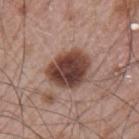This lesion was catalogued during total-body skin photography and was not selected for biopsy. This is a white-light tile. The lesion is located on the arm. The total-body-photography lesion software estimated a footprint of about 16 mm², an eccentricity of roughly 0.6, and a shape-asymmetry score of about 0.15 (0 = symmetric). The software also gave a mean CIELAB color near L≈41 a*≈20 b*≈24, a lesion–skin lightness drop of about 18, and a lesion-to-skin contrast of about 13 (normalized; higher = more distinct). The analysis additionally found a nevus-likeness score of about 35/100. A 15 mm close-up tile from a total-body photography series done for melanoma screening. A male patient, aged approximately 65. Longest diameter approximately 5 mm.